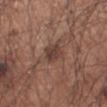| feature | finding |
|---|---|
| biopsy status | no biopsy performed (imaged during a skin exam) |
| lesion size | ~3 mm (longest diameter) |
| subject | male, aged around 55 |
| acquisition | total-body-photography crop, ~15 mm field of view |
| automated lesion analysis | a mean CIELAB color near L≈40 a*≈18 b*≈23, a lesion–skin lightness drop of about 9, and a normalized lesion–skin contrast near 7.5; an automated nevus-likeness rating near 25 out of 100 and a detector confidence of about 100 out of 100 that the crop contains a lesion |
| tile lighting | white-light illumination |
| body site | the left forearm |